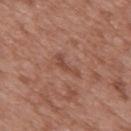| feature | finding |
|---|---|
| workup | no biopsy performed (imaged during a skin exam) |
| subject | male, approximately 50 years of age |
| diameter | ~3.5 mm (longest diameter) |
| image source | ~15 mm crop, total-body skin-cancer survey |
| body site | the mid back |
| tile lighting | white-light illumination |